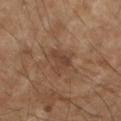Impression:
Recorded during total-body skin imaging; not selected for excision or biopsy.
Clinical summary:
Imaged with cross-polarized lighting. A female subject in their 60s. A 15 mm crop from a total-body photograph taken for skin-cancer surveillance. Located on the right forearm.